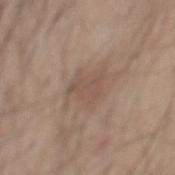Captured during whole-body skin photography for melanoma surveillance; the lesion was not biopsied.
An algorithmic analysis of the crop reported a lesion color around L≈51 a*≈16 b*≈25 in CIELAB, about 6 CIELAB-L* units darker than the surrounding skin, and a normalized lesion–skin contrast near 5. And it measured a border-irregularity index near 5/10, internal color variation of about 2.5 on a 0–10 scale, and peripheral color asymmetry of about 1. The software also gave a detector confidence of about 100 out of 100 that the crop contains a lesion.
A male subject roughly 60 years of age.
A close-up tile cropped from a whole-body skin photograph, about 15 mm across.
On the mid back.